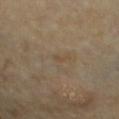Q: Was a biopsy performed?
A: no biopsy performed (imaged during a skin exam)
Q: What lighting was used for the tile?
A: cross-polarized
Q: Where on the body is the lesion?
A: the chest
Q: What kind of image is this?
A: ~15 mm tile from a whole-body skin photo
Q: Patient demographics?
A: female, roughly 60 years of age
Q: What did automated image analysis measure?
A: a footprint of about 0.5 mm², an outline eccentricity of about 0.85 (0 = round, 1 = elongated), and a symmetry-axis asymmetry near 0.4; a border-irregularity index near 3/10, a within-lesion color-variation index near 0/10, and a peripheral color-asymmetry measure near 0